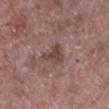<record>
<biopsy_status>not biopsied; imaged during a skin examination</biopsy_status>
<image>
  <source>total-body photography crop</source>
  <field_of_view_mm>15</field_of_view_mm>
</image>
<patient>
  <sex>male</sex>
  <age_approx>55</age_approx>
</patient>
<site>right lower leg</site>
</record>